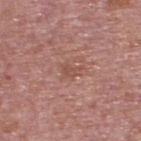{
  "biopsy_status": "not biopsied; imaged during a skin examination",
  "patient": {
    "sex": "male",
    "age_approx": 75
  },
  "lighting": "white-light",
  "site": "upper back",
  "lesion_size": {
    "long_diameter_mm_approx": 2.5
  },
  "automated_metrics": {
    "cielab_L": 51,
    "cielab_a": 23,
    "cielab_b": 26,
    "vs_skin_darker_L": 7.0,
    "vs_skin_contrast_norm": 5.0,
    "border_irregularity_0_10": 4.0,
    "color_variation_0_10": 1.5,
    "peripheral_color_asymmetry": 0.5,
    "lesion_detection_confidence_0_100": 100
  },
  "image": {
    "source": "total-body photography crop",
    "field_of_view_mm": 15
  }
}